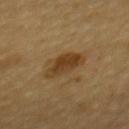This lesion was catalogued during total-body skin photography and was not selected for biopsy. The tile uses cross-polarized illumination. Located on the back. Cropped from a whole-body photographic skin survey; the tile spans about 15 mm. A female subject aged around 50. About 4.5 mm across.